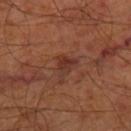A patient aged approximately 65. The lesion-visualizer software estimated an area of roughly 5 mm² and two-axis asymmetry of about 0.45. The software also gave a lesion color around L≈33 a*≈23 b*≈26 in CIELAB. The analysis additionally found a border-irregularity rating of about 4/10. A 15 mm crop from a total-body photograph taken for skin-cancer surveillance. This is a cross-polarized tile. On the right thigh.